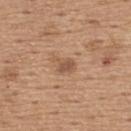Case summary:
* lesion diameter · ≈3 mm
* image source · 15 mm crop, total-body photography
* tile lighting · white-light
* TBP lesion metrics · a footprint of about 4 mm² and a shape eccentricity near 0.7; a mean CIELAB color near L≈54 a*≈20 b*≈31 and about 9 CIELAB-L* units darker than the surrounding skin
* site · the upper back
* subject · male, aged 63–67